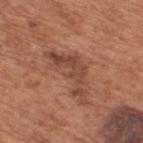Impression: Imaged during a routine full-body skin examination; the lesion was not biopsied and no histopathology is available. Background: The lesion is on the upper back. The patient is a male about 65 years old. A region of skin cropped from a whole-body photographic capture, roughly 15 mm wide.